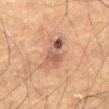workup: imaged on a skin check; not biopsied | patient: male, in their mid- to late 50s | anatomic site: the front of the torso | imaging modality: ~15 mm crop, total-body skin-cancer survey.